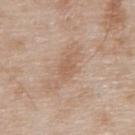{
  "biopsy_status": "not biopsied; imaged during a skin examination",
  "automated_metrics": {
    "area_mm2_approx": 3.0,
    "eccentricity": 0.75,
    "shape_asymmetry": 0.3,
    "nevus_likeness_0_100": 0
  },
  "patient": {
    "sex": "male",
    "age_approx": 80
  },
  "lighting": "white-light",
  "image": {
    "source": "total-body photography crop",
    "field_of_view_mm": 15
  },
  "lesion_size": {
    "long_diameter_mm_approx": 2.5
  },
  "site": "chest"
}